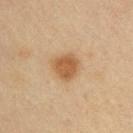| feature | finding |
|---|---|
| notes | total-body-photography surveillance lesion; no biopsy |
| automated metrics | a border-irregularity rating of about 2.5/10 |
| body site | the upper back |
| tile lighting | cross-polarized illumination |
| subject | male, aged 33 to 37 |
| diameter | about 3 mm |
| image source | ~15 mm crop, total-body skin-cancer survey |